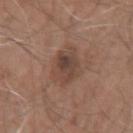Image and clinical context: The total-body-photography lesion software estimated a footprint of about 11 mm² and a symmetry-axis asymmetry near 0.2. And it measured a border-irregularity rating of about 2.5/10, a color-variation rating of about 5.5/10, and peripheral color asymmetry of about 2. The software also gave a detector confidence of about 100 out of 100 that the crop contains a lesion. This is a white-light tile. This image is a 15 mm lesion crop taken from a total-body photograph. From the arm. A male subject in their 60s. The lesion's longest dimension is about 4 mm.